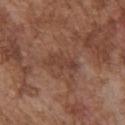The lesion was tiled from a total-body skin photograph and was not biopsied. The tile uses white-light illumination. The recorded lesion diameter is about 4 mm. The patient is a male roughly 75 years of age. Automated image analysis of the tile measured a border-irregularity index near 3.5/10, internal color variation of about 1.5 on a 0–10 scale, and a peripheral color-asymmetry measure near 0.5. From the front of the torso. A 15 mm close-up tile from a total-body photography series done for melanoma screening.